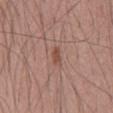This lesion was catalogued during total-body skin photography and was not selected for biopsy.
The total-body-photography lesion software estimated an area of roughly 3 mm², an outline eccentricity of about 0.85 (0 = round, 1 = elongated), and a shape-asymmetry score of about 0.3 (0 = symmetric). And it measured an average lesion color of about L≈50 a*≈21 b*≈26 (CIELAB) and a lesion-to-skin contrast of about 6.5 (normalized; higher = more distinct). The analysis additionally found a border-irregularity rating of about 3/10 and a within-lesion color-variation index near 1.5/10. The software also gave a nevus-likeness score of about 55/100.
Cropped from a total-body skin-imaging series; the visible field is about 15 mm.
The recorded lesion diameter is about 2.5 mm.
Located on the chest.
The tile uses white-light illumination.
The subject is a male aged 38 to 42.